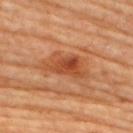Q: Was a biopsy performed?
A: no biopsy performed (imaged during a skin exam)
Q: Patient demographics?
A: female, roughly 65 years of age
Q: Where on the body is the lesion?
A: the upper back
Q: What kind of image is this?
A: total-body-photography crop, ~15 mm field of view